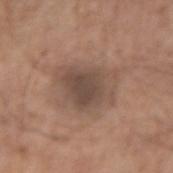| feature | finding |
|---|---|
| biopsy status | imaged on a skin check; not biopsied |
| body site | the right forearm |
| image source | ~15 mm tile from a whole-body skin photo |
| illumination | white-light |
| subject | male, in their mid-60s |